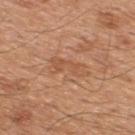biopsy_status: not biopsied; imaged during a skin examination
lighting: white-light
site: upper back
patient:
  sex: male
  age_approx: 45
automated_metrics:
  border_irregularity_0_10: 6.0
  color_variation_0_10: 1.5
  peripheral_color_asymmetry: 0.5
lesion_size:
  long_diameter_mm_approx: 4.5
image:
  source: total-body photography crop
  field_of_view_mm: 15